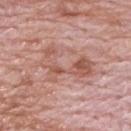This is a white-light tile.
Located on the upper back.
Automated image analysis of the tile measured a footprint of about 13 mm², an outline eccentricity of about 0.85 (0 = round, 1 = elongated), and a symmetry-axis asymmetry near 0.55. The software also gave a border-irregularity index near 9/10, a color-variation rating of about 5.5/10, and a peripheral color-asymmetry measure near 2.5. It also reported an automated nevus-likeness rating near 0 out of 100 and a lesion-detection confidence of about 100/100.
About 6 mm across.
The patient is a male aged 68 to 72.
A lesion tile, about 15 mm wide, cut from a 3D total-body photograph.